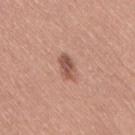Part of a total-body skin-imaging series; this lesion was reviewed on a skin check and was not flagged for biopsy.
On the right thigh.
A roughly 15 mm field-of-view crop from a total-body skin photograph.
The recorded lesion diameter is about 3.5 mm.
This is a white-light tile.
The subject is a female approximately 50 years of age.
The lesion-visualizer software estimated a normalized lesion–skin contrast near 8. And it measured a nevus-likeness score of about 90/100 and a lesion-detection confidence of about 100/100.